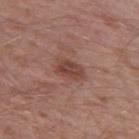workup = total-body-photography surveillance lesion; no biopsy | patient = male, about 60 years old | acquisition = ~15 mm tile from a whole-body skin photo | location = the leg | diameter = about 4 mm.